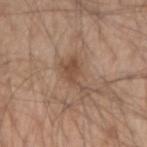Q: Was a biopsy performed?
A: no biopsy performed (imaged during a skin exam)